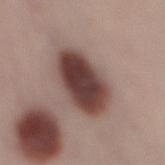follow-up: catalogued during a skin exam; not biopsied | subject: female, aged 23–27 | body site: the leg | image: total-body-photography crop, ~15 mm field of view.